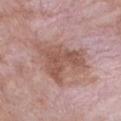Q: Is there a histopathology result?
A: total-body-photography surveillance lesion; no biopsy
Q: Automated lesion metrics?
A: a mean CIELAB color near L≈54 a*≈21 b*≈25, about 10 CIELAB-L* units darker than the surrounding skin, and a normalized lesion–skin contrast near 7.5; a classifier nevus-likeness of about 0/100
Q: How large is the lesion?
A: ≈7 mm
Q: Who is the patient?
A: male, in their 50s
Q: How was the tile lit?
A: white-light
Q: How was this image acquired?
A: 15 mm crop, total-body photography
Q: What is the anatomic site?
A: the front of the torso